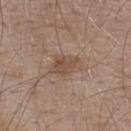Clinical impression:
No biopsy was performed on this lesion — it was imaged during a full skin examination and was not determined to be concerning.
Clinical summary:
The subject is a male in their mid- to late 50s. A region of skin cropped from a whole-body photographic capture, roughly 15 mm wide. The lesion is on the upper back.